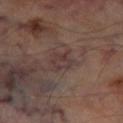Recorded during total-body skin imaging; not selected for excision or biopsy. The lesion-visualizer software estimated a within-lesion color-variation index near 3/10 and peripheral color asymmetry of about 1. And it measured lesion-presence confidence of about 90/100. This image is a 15 mm lesion crop taken from a total-body photograph. Captured under cross-polarized illumination. From the left thigh. A male subject, aged 68 to 72.